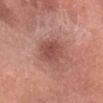Image and clinical context:
A female patient, aged around 55. Automated image analysis of the tile measured a lesion color around L≈49 a*≈25 b*≈25 in CIELAB. Cropped from a whole-body photographic skin survey; the tile spans about 15 mm. The lesion is on the head or neck.